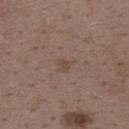Part of a total-body skin-imaging series; this lesion was reviewed on a skin check and was not flagged for biopsy. On the upper back. Measured at roughly 3 mm in maximum diameter. A lesion tile, about 15 mm wide, cut from a 3D total-body photograph. A female patient aged around 35.